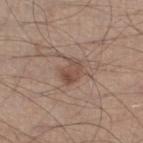Clinical impression: The lesion was tiled from a total-body skin photograph and was not biopsied. Image and clinical context: Located on the left lower leg. Longest diameter approximately 3 mm. Cropped from a whole-body photographic skin survey; the tile spans about 15 mm. Imaged with white-light lighting. The lesion-visualizer software estimated an eccentricity of roughly 0.75 and two-axis asymmetry of about 0.3. And it measured an average lesion color of about L≈48 a*≈18 b*≈25 (CIELAB) and a normalized border contrast of about 6.5. It also reported an automated nevus-likeness rating near 75 out of 100 and lesion-presence confidence of about 100/100. The patient is a male in their mid-50s.